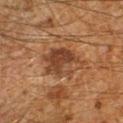biopsy status — catalogued during a skin exam; not biopsied
image-analysis metrics — a lesion area of about 13 mm², an eccentricity of roughly 0.6, and two-axis asymmetry of about 0.2; a border-irregularity rating of about 2.5/10, internal color variation of about 6 on a 0–10 scale, and radial color variation of about 2
patient — male, aged around 60
illumination — cross-polarized
image source — ~15 mm crop, total-body skin-cancer survey
diameter — ~5 mm (longest diameter)
body site — the left forearm